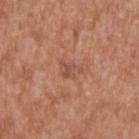{
  "biopsy_status": "not biopsied; imaged during a skin examination",
  "lighting": "white-light",
  "image": {
    "source": "total-body photography crop",
    "field_of_view_mm": 15
  },
  "automated_metrics": {
    "area_mm2_approx": 3.5,
    "eccentricity": 0.75,
    "shape_asymmetry": 0.4,
    "cielab_L": 51,
    "cielab_a": 24,
    "cielab_b": 29,
    "vs_skin_darker_L": 7.0,
    "vs_skin_contrast_norm": 5.0,
    "border_irregularity_0_10": 5.0,
    "color_variation_0_10": 1.0,
    "nevus_likeness_0_100": 0
  },
  "site": "upper back",
  "lesion_size": {
    "long_diameter_mm_approx": 3.0
  },
  "patient": {
    "sex": "male",
    "age_approx": 65
  }
}